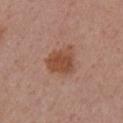The recorded lesion diameter is about 4 mm.
This image is a 15 mm lesion crop taken from a total-body photograph.
A female patient, about 55 years old.
The lesion is located on the right upper arm.
Automated tile analysis of the lesion measured an area of roughly 9 mm² and a shape eccentricity near 0.5. The software also gave a mean CIELAB color near L≈48 a*≈23 b*≈31, a lesion–skin lightness drop of about 10, and a lesion-to-skin contrast of about 8.5 (normalized; higher = more distinct). And it measured a border-irregularity index near 3/10, a color-variation rating of about 2.5/10, and peripheral color asymmetry of about 1.